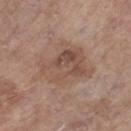The lesion was photographed on a routine skin check and not biopsied; there is no pathology result. This is a white-light tile. On the right lower leg. A region of skin cropped from a whole-body photographic capture, roughly 15 mm wide. Measured at roughly 6 mm in maximum diameter. The subject is a female aged around 85 or older.